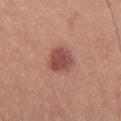Clinical impression:
This lesion was catalogued during total-body skin photography and was not selected for biopsy.
Background:
An algorithmic analysis of the crop reported an area of roughly 8 mm² and a shape eccentricity near 0.2. It also reported about 12 CIELAB-L* units darker than the surrounding skin and a normalized lesion–skin contrast near 8.5. The software also gave a within-lesion color-variation index near 3.5/10 and a peripheral color-asymmetry measure near 1.5. Cropped from a total-body skin-imaging series; the visible field is about 15 mm. From the upper back. Approximately 3 mm at its widest. A female patient aged approximately 35.